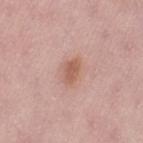Assessment: This lesion was catalogued during total-body skin photography and was not selected for biopsy. Image and clinical context: The lesion is located on the lower back. The total-body-photography lesion software estimated a shape eccentricity near 0.8 and a shape-asymmetry score of about 0.2 (0 = symmetric). The software also gave a peripheral color-asymmetry measure near 0.5. Cropped from a whole-body photographic skin survey; the tile spans about 15 mm. A female patient approximately 50 years of age.